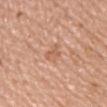follow-up=imaged on a skin check; not biopsied | location=the chest | subject=female, aged approximately 45 | lesion diameter=about 2.5 mm | imaging modality=15 mm crop, total-body photography | lighting=white-light illumination.